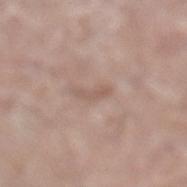  biopsy_status: not biopsied; imaged during a skin examination
  image:
    source: total-body photography crop
    field_of_view_mm: 15
  site: left lower leg
  automated_metrics:
    area_mm2_approx: 2.5
    eccentricity: 0.95
    shape_asymmetry: 0.5
    cielab_L: 56
    cielab_a: 17
    cielab_b: 24
    vs_skin_darker_L: 7.0
    vs_skin_contrast_norm: 5.0
  patient:
    sex: male
    age_approx: 70
  lesion_size:
    long_diameter_mm_approx: 3.0